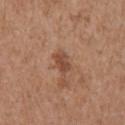A close-up tile cropped from a whole-body skin photograph, about 15 mm across. The total-body-photography lesion software estimated a shape eccentricity near 0.8 and two-axis asymmetry of about 0.3. The software also gave a nevus-likeness score of about 5/100. A female patient about 75 years old. The recorded lesion diameter is about 3 mm. The tile uses white-light illumination. The lesion is located on the right upper arm.